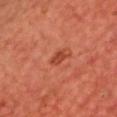Clinical impression: Captured during whole-body skin photography for melanoma surveillance; the lesion was not biopsied. Background: The patient is a male approximately 65 years of age. The lesion is on the chest. A lesion tile, about 15 mm wide, cut from a 3D total-body photograph. An algorithmic analysis of the crop reported a lesion area of about 3 mm² and a shape eccentricity near 0.75. And it measured a mean CIELAB color near L≈45 a*≈33 b*≈36 and roughly 9 lightness units darker than nearby skin. It also reported a border-irregularity rating of about 2/10 and a color-variation rating of about 2/10. The software also gave lesion-presence confidence of about 100/100.